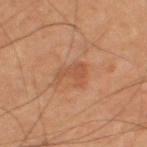| feature | finding |
|---|---|
| follow-up | no biopsy performed (imaged during a skin exam) |
| TBP lesion metrics | an outline eccentricity of about 0.7 (0 = round, 1 = elongated) and a shape-asymmetry score of about 0.6 (0 = symmetric); an average lesion color of about L≈46 a*≈22 b*≈31 (CIELAB), about 6 CIELAB-L* units darker than the surrounding skin, and a normalized lesion–skin contrast near 5; a classifier nevus-likeness of about 10/100 and lesion-presence confidence of about 100/100 |
| lighting | cross-polarized illumination |
| diameter | ≈3 mm |
| acquisition | 15 mm crop, total-body photography |
| body site | the right thigh |
| patient | male, about 60 years old |